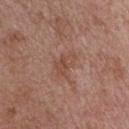Image and clinical context:
Measured at roughly 3.5 mm in maximum diameter. An algorithmic analysis of the crop reported a lesion–skin lightness drop of about 7. The software also gave an automated nevus-likeness rating near 0 out of 100 and a detector confidence of about 100 out of 100 that the crop contains a lesion. The tile uses white-light illumination. Cropped from a total-body skin-imaging series; the visible field is about 15 mm. On the chest. The patient is a male in their mid- to late 60s.